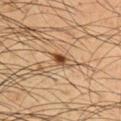No biopsy was performed on this lesion — it was imaged during a full skin examination and was not determined to be concerning. A roughly 15 mm field-of-view crop from a total-body skin photograph. Measured at roughly 2.5 mm in maximum diameter. The lesion is located on the upper back. A male subject aged 48 to 52.